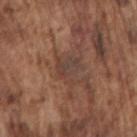- workup · catalogued during a skin exam; not biopsied
- imaging modality · 15 mm crop, total-body photography
- size · ~3.5 mm (longest diameter)
- illumination · white-light
- patient · male, in their mid-70s
- body site · the right upper arm